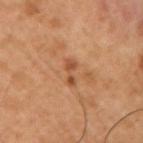  biopsy_status: not biopsied; imaged during a skin examination
  lighting: cross-polarized
  site: right upper arm
  patient:
    sex: male
    age_approx: 50
  image:
    source: total-body photography crop
    field_of_view_mm: 15
  lesion_size:
    long_diameter_mm_approx: 3.0